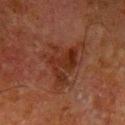Clinical impression: The lesion was photographed on a routine skin check and not biopsied; there is no pathology result. Background: Automated image analysis of the tile measured a mean CIELAB color near L≈24 a*≈20 b*≈24, a lesion–skin lightness drop of about 6, and a lesion-to-skin contrast of about 7.5 (normalized; higher = more distinct). The tile uses cross-polarized illumination. The lesion is located on the arm. A lesion tile, about 15 mm wide, cut from a 3D total-body photograph. The subject is a male approximately 80 years of age.